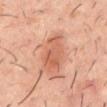Findings:
- biopsy status — total-body-photography surveillance lesion; no biopsy
- patient — male, about 60 years old
- imaging modality — ~15 mm crop, total-body skin-cancer survey
- anatomic site — the back
- lesion size — about 6.5 mm